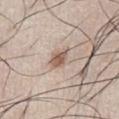Recorded during total-body skin imaging; not selected for excision or biopsy. A male patient, aged around 45. Located on the chest. A roughly 15 mm field-of-view crop from a total-body skin photograph.